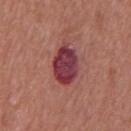| field | value |
|---|---|
| follow-up | total-body-photography surveillance lesion; no biopsy |
| diameter | ~5 mm (longest diameter) |
| image | ~15 mm crop, total-body skin-cancer survey |
| illumination | white-light |
| subject | male, aged around 65 |
| location | the chest |